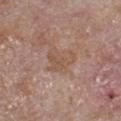Captured during whole-body skin photography for melanoma surveillance; the lesion was not biopsied. The lesion is on the chest. Captured under white-light illumination. A lesion tile, about 15 mm wide, cut from a 3D total-body photograph. A male patient in their mid- to late 60s.